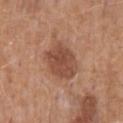  biopsy_status: not biopsied; imaged during a skin examination
  automated_metrics:
    area_mm2_approx: 14.0
    eccentricity: 0.7
    shape_asymmetry: 0.15
    cielab_L: 48
    cielab_a: 23
    cielab_b: 29
    vs_skin_contrast_norm: 8.0
    color_variation_0_10: 3.0
    peripheral_color_asymmetry: 1.0
  site: mid back
  lighting: white-light
  patient:
    sex: male
    age_approx: 70
  lesion_size:
    long_diameter_mm_approx: 5.0
  image:
    source: total-body photography crop
    field_of_view_mm: 15